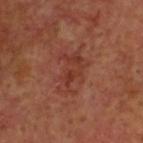Clinical impression: This lesion was catalogued during total-body skin photography and was not selected for biopsy. Acquisition and patient details: A male patient in their 60s. A region of skin cropped from a whole-body photographic capture, roughly 15 mm wide. The lesion is located on the head or neck. An algorithmic analysis of the crop reported a footprint of about 12 mm², a shape eccentricity near 0.8, and a symmetry-axis asymmetry near 0.4. The analysis additionally found a border-irregularity rating of about 7/10, a color-variation rating of about 3.5/10, and peripheral color asymmetry of about 1. The analysis additionally found a nevus-likeness score of about 0/100 and a detector confidence of about 100 out of 100 that the crop contains a lesion. Captured under cross-polarized illumination. Measured at roughly 5 mm in maximum diameter.